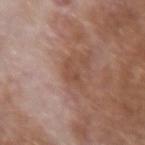| field | value |
|---|---|
| follow-up | imaged on a skin check; not biopsied |
| diameter | about 2.5 mm |
| image-analysis metrics | a footprint of about 3.5 mm², a shape eccentricity near 0.8, and a symmetry-axis asymmetry near 0.45; border irregularity of about 5 on a 0–10 scale, internal color variation of about 1.5 on a 0–10 scale, and peripheral color asymmetry of about 0.5 |
| location | the right forearm |
| acquisition | ~15 mm crop, total-body skin-cancer survey |
| patient | male, in their mid- to late 60s |